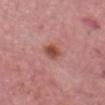This lesion was catalogued during total-body skin photography and was not selected for biopsy.
From the head or neck.
A male patient aged 63 to 67.
A 15 mm crop from a total-body photograph taken for skin-cancer surveillance.